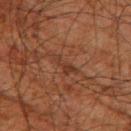follow-up: total-body-photography surveillance lesion; no biopsy | image source: ~15 mm crop, total-body skin-cancer survey | subject: male, about 80 years old | location: the left thigh | lighting: cross-polarized illumination.